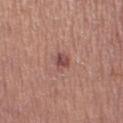lesion diameter: ≈2.5 mm
lighting: white-light
location: the right thigh
patient: female, in their mid-20s
automated lesion analysis: a color-variation rating of about 3/10 and peripheral color asymmetry of about 1; a classifier nevus-likeness of about 65/100 and a detector confidence of about 100 out of 100 that the crop contains a lesion
acquisition: ~15 mm crop, total-body skin-cancer survey